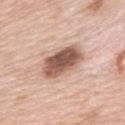imaging modality: 15 mm crop, total-body photography; patient: female, in their mid-60s; location: the upper back.